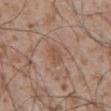<lesion>
  <biopsy_status>not biopsied; imaged during a skin examination</biopsy_status>
  <lesion_size>
    <long_diameter_mm_approx>2.5</long_diameter_mm_approx>
  </lesion_size>
  <image>
    <source>total-body photography crop</source>
    <field_of_view_mm>15</field_of_view_mm>
  </image>
  <patient>
    <sex>male</sex>
    <age_approx>55</age_approx>
  </patient>
  <site>abdomen</site>
  <automated_metrics>
    <border_irregularity_0_10>2.0</border_irregularity_0_10>
    <color_variation_0_10>1.5</color_variation_0_10>
    <peripheral_color_asymmetry>0.5</peripheral_color_asymmetry>
  </automated_metrics>
</lesion>